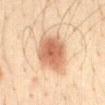Imaged during a routine full-body skin examination; the lesion was not biopsied and no histopathology is available.
Approximately 5 mm at its widest.
A roughly 15 mm field-of-view crop from a total-body skin photograph.
A male patient, aged approximately 35.
From the front of the torso.
Automated tile analysis of the lesion measured an area of roughly 17 mm², an outline eccentricity of about 0.5 (0 = round, 1 = elongated), and a shape-asymmetry score of about 0.15 (0 = symmetric).
The tile uses cross-polarized illumination.